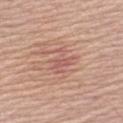Part of a total-body skin-imaging series; this lesion was reviewed on a skin check and was not flagged for biopsy.
The lesion is located on the left upper arm.
This image is a 15 mm lesion crop taken from a total-body photograph.
About 3.5 mm across.
A male subject approximately 65 years of age.